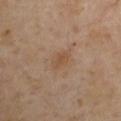* workup · no biopsy performed (imaged during a skin exam)
* anatomic site · the right upper arm
* patient · male, roughly 55 years of age
* imaging modality · total-body-photography crop, ~15 mm field of view